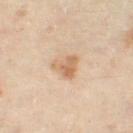| key | value |
|---|---|
| workup | imaged on a skin check; not biopsied |